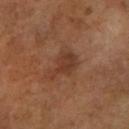Impression:
No biopsy was performed on this lesion — it was imaged during a full skin examination and was not determined to be concerning.
Image and clinical context:
Approximately 4 mm at its widest. A female subject approximately 60 years of age. Located on the left forearm. A close-up tile cropped from a whole-body skin photograph, about 15 mm across.